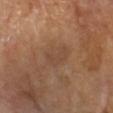The lesion was tiled from a total-body skin photograph and was not biopsied. Cropped from a whole-body photographic skin survey; the tile spans about 15 mm. Longest diameter approximately 4.5 mm. A female subject, aged 53 to 57. This is a cross-polarized tile. The lesion is located on the left forearm.